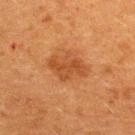  biopsy_status: not biopsied; imaged during a skin examination
  patient:
    sex: female
    age_approx: 40
  site: back
  automated_metrics:
    area_mm2_approx: 9.5
    shape_asymmetry: 0.2
    lesion_detection_confidence_0_100: 100
  lighting: cross-polarized
  image:
    source: total-body photography crop
    field_of_view_mm: 15
  lesion_size:
    long_diameter_mm_approx: 4.5